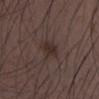A lesion tile, about 15 mm wide, cut from a 3D total-body photograph. The patient is a male in their 30s. From the chest. The lesion's longest dimension is about 2.5 mm. This is a white-light tile.